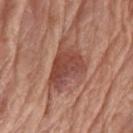Assessment: Captured during whole-body skin photography for melanoma surveillance; the lesion was not biopsied. Context: The tile uses white-light illumination. The lesion's longest dimension is about 6 mm. The lesion-visualizer software estimated a border-irregularity index near 5.5/10, a color-variation rating of about 5/10, and radial color variation of about 1.5. And it measured a nevus-likeness score of about 45/100 and a lesion-detection confidence of about 100/100. A female subject, aged approximately 75. A close-up tile cropped from a whole-body skin photograph, about 15 mm across. The lesion is on the left upper arm.